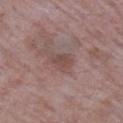{
  "image": {
    "source": "total-body photography crop",
    "field_of_view_mm": 15
  },
  "site": "arm",
  "patient": {
    "sex": "male",
    "age_approx": 65
  }
}